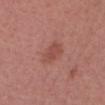Impression: The lesion was photographed on a routine skin check and not biopsied; there is no pathology result. Acquisition and patient details: The lesion is located on the head or neck. A region of skin cropped from a whole-body photographic capture, roughly 15 mm wide. Imaged with white-light lighting. The recorded lesion diameter is about 3 mm. The subject is a female aged approximately 55.